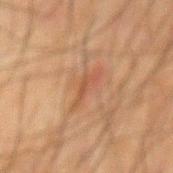body site: the back
subject: male, aged approximately 65
illumination: cross-polarized illumination
acquisition: total-body-photography crop, ~15 mm field of view